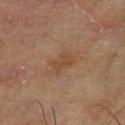notes: catalogued during a skin exam; not biopsied | image: ~15 mm tile from a whole-body skin photo | lesion size: ~2.5 mm (longest diameter) | patient: male, aged 63 to 67 | body site: the left lower leg | tile lighting: cross-polarized.